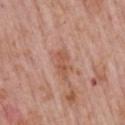follow-up: imaged on a skin check; not biopsied | body site: the mid back | automated lesion analysis: a footprint of about 3 mm², an outline eccentricity of about 0.9 (0 = round, 1 = elongated), and two-axis asymmetry of about 0.45; a border-irregularity rating of about 5/10, internal color variation of about 0 on a 0–10 scale, and a peripheral color-asymmetry measure near 0; a nevus-likeness score of about 0/100 and a detector confidence of about 100 out of 100 that the crop contains a lesion | image source: total-body-photography crop, ~15 mm field of view | lighting: white-light illumination | subject: male, in their mid-70s | size: about 3 mm.